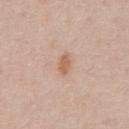Clinical impression:
No biopsy was performed on this lesion — it was imaged during a full skin examination and was not determined to be concerning.
Image and clinical context:
Automated image analysis of the tile measured a footprint of about 3.5 mm², a shape eccentricity near 0.8, and a shape-asymmetry score of about 0.2 (0 = symmetric). The analysis additionally found an automated nevus-likeness rating near 40 out of 100 and lesion-presence confidence of about 100/100. Located on the abdomen. The tile uses white-light illumination. A male subject, aged around 60. Cropped from a total-body skin-imaging series; the visible field is about 15 mm. The recorded lesion diameter is about 2.5 mm.